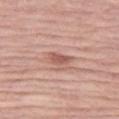The lesion was tiled from a total-body skin photograph and was not biopsied.
Captured under white-light illumination.
The subject is a female aged around 70.
Automated image analysis of the tile measured a lesion area of about 4 mm², an outline eccentricity of about 0.85 (0 = round, 1 = elongated), and a shape-asymmetry score of about 0.35 (0 = symmetric).
Measured at roughly 3 mm in maximum diameter.
On the left thigh.
Cropped from a whole-body photographic skin survey; the tile spans about 15 mm.